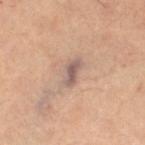Located on the right thigh. This image is a 15 mm lesion crop taken from a total-body photograph. A female patient, roughly 40 years of age. The lesion's longest dimension is about 3.5 mm. Automated image analysis of the tile measured lesion-presence confidence of about 90/100.